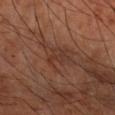patient: male, approximately 60 years of age
lighting: cross-polarized
TBP lesion metrics: a lesion area of about 2.5 mm², an eccentricity of roughly 0.9, and a shape-asymmetry score of about 0.5 (0 = symmetric); border irregularity of about 6 on a 0–10 scale, internal color variation of about 0 on a 0–10 scale, and radial color variation of about 0; a nevus-likeness score of about 0/100 and a lesion-detection confidence of about 70/100
acquisition: total-body-photography crop, ~15 mm field of view
anatomic site: the right forearm